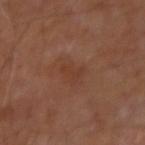Notes:
- biopsy status: catalogued during a skin exam; not biopsied
- location: the left arm
- illumination: cross-polarized illumination
- acquisition: 15 mm crop, total-body photography
- subject: male, approximately 50 years of age
- size: about 2.5 mm
- automated lesion analysis: an area of roughly 4 mm², a shape eccentricity near 0.7, and two-axis asymmetry of about 0.45; a lesion–skin lightness drop of about 4 and a normalized lesion–skin contrast near 4.5